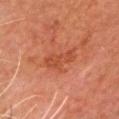Q: Was a biopsy performed?
A: catalogued during a skin exam; not biopsied
Q: Who is the patient?
A: male, aged 78–82
Q: How was this image acquired?
A: ~15 mm crop, total-body skin-cancer survey
Q: Lesion size?
A: about 4 mm
Q: How was the tile lit?
A: cross-polarized illumination
Q: Where on the body is the lesion?
A: the head or neck